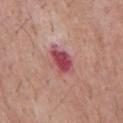biopsy status: imaged on a skin check; not biopsied
diameter: ≈4.5 mm
location: the chest
subject: male, aged around 55
imaging modality: ~15 mm tile from a whole-body skin photo